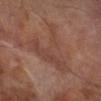Q: Was a biopsy performed?
A: catalogued during a skin exam; not biopsied
Q: Illumination type?
A: cross-polarized illumination
Q: What did automated image analysis measure?
A: a footprint of about 18 mm², a shape eccentricity near 0.7, and a shape-asymmetry score of about 0.8 (0 = symmetric)
Q: Lesion location?
A: the right lower leg
Q: What is the imaging modality?
A: ~15 mm tile from a whole-body skin photo
Q: What is the lesion's diameter?
A: ~7.5 mm (longest diameter)
Q: What are the patient's age and sex?
A: male, aged around 70